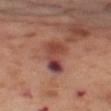Assessment: The lesion was tiled from a total-body skin photograph and was not biopsied. Background: A female patient aged 53–57. Measured at roughly 4.5 mm in maximum diameter. An algorithmic analysis of the crop reported an area of roughly 10 mm² and a shape-asymmetry score of about 0.4 (0 = symmetric). And it measured a mean CIELAB color near L≈44 a*≈26 b*≈25. It also reported a nevus-likeness score of about 0/100. A 15 mm crop from a total-body photograph taken for skin-cancer surveillance. Imaged with cross-polarized lighting. From the left thigh.